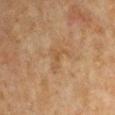{"biopsy_status": "not biopsied; imaged during a skin examination", "patient": {"sex": "male", "age_approx": 50}, "site": "left upper arm", "automated_metrics": {"area_mm2_approx": 4.0, "eccentricity": 0.8, "shape_asymmetry": 0.7}, "image": {"source": "total-body photography crop", "field_of_view_mm": 15}}